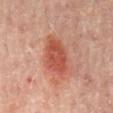Clinical impression: Captured during whole-body skin photography for melanoma surveillance; the lesion was not biopsied. Image and clinical context: A male patient, aged around 65. Automated tile analysis of the lesion measured a lesion area of about 13 mm², a shape eccentricity near 0.85, and a shape-asymmetry score of about 0.2 (0 = symmetric). The software also gave a classifier nevus-likeness of about 100/100 and lesion-presence confidence of about 100/100. A close-up tile cropped from a whole-body skin photograph, about 15 mm across. On the mid back.